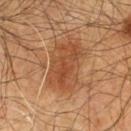Impression: Recorded during total-body skin imaging; not selected for excision or biopsy. Acquisition and patient details: From the chest. The subject is a male in their 60s. Cropped from a whole-body photographic skin survey; the tile spans about 15 mm.